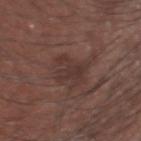Q: Was this lesion biopsied?
A: imaged on a skin check; not biopsied
Q: Who is the patient?
A: male, aged approximately 35
Q: What did automated image analysis measure?
A: about 6 CIELAB-L* units darker than the surrounding skin and a normalized lesion–skin contrast near 6; a border-irregularity index near 6.5/10; a nevus-likeness score of about 0/100 and a lesion-detection confidence of about 100/100
Q: What kind of image is this?
A: ~15 mm tile from a whole-body skin photo
Q: What lighting was used for the tile?
A: white-light illumination
Q: What is the anatomic site?
A: the head or neck